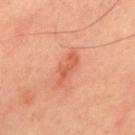biopsy status — total-body-photography surveillance lesion; no biopsy | patient — male, aged 48–52 | size — ≈4 mm | illumination — cross-polarized illumination | body site — the upper back | image source — 15 mm crop, total-body photography | automated metrics — two-axis asymmetry of about 0.4; a lesion color around L≈59 a*≈32 b*≈37 in CIELAB, a lesion–skin lightness drop of about 9, and a lesion-to-skin contrast of about 6.5 (normalized; higher = more distinct); border irregularity of about 5 on a 0–10 scale.